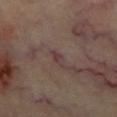biopsy status: imaged on a skin check; not biopsied
size: about 3 mm
imaging modality: ~15 mm tile from a whole-body skin photo
automated metrics: an area of roughly 2.5 mm², an eccentricity of roughly 0.95, and a shape-asymmetry score of about 0.25 (0 = symmetric); a nevus-likeness score of about 0/100 and lesion-presence confidence of about 70/100
body site: the chest